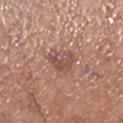follow-up = no biopsy performed (imaged during a skin exam)
acquisition = total-body-photography crop, ~15 mm field of view
patient = male, aged around 70
site = the right lower leg
lighting = white-light
lesion size = ~3.5 mm (longest diameter)